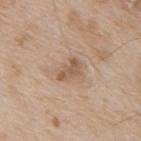follow-up: total-body-photography surveillance lesion; no biopsy
TBP lesion metrics: a footprint of about 4 mm², an outline eccentricity of about 0.85 (0 = round, 1 = elongated), and two-axis asymmetry of about 0.35; an average lesion color of about L≈55 a*≈17 b*≈30 (CIELAB) and a lesion-to-skin contrast of about 6.5 (normalized; higher = more distinct)
lighting: white-light illumination
anatomic site: the upper back
subject: male, roughly 65 years of age
image: ~15 mm tile from a whole-body skin photo
lesion size: ~3 mm (longest diameter)